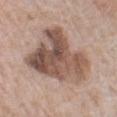Part of a total-body skin-imaging series; this lesion was reviewed on a skin check and was not flagged for biopsy.
Captured under white-light illumination.
Cropped from a whole-body photographic skin survey; the tile spans about 15 mm.
On the left lower leg.
A female patient aged 73 to 77.